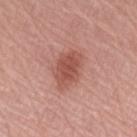Recorded during total-body skin imaging; not selected for excision or biopsy. A region of skin cropped from a whole-body photographic capture, roughly 15 mm wide. A female subject, in their mid-60s. On the right forearm. Imaged with white-light lighting. About 4.5 mm across.